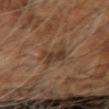{
  "biopsy_status": "not biopsied; imaged during a skin examination",
  "lighting": "cross-polarized",
  "image": {
    "source": "total-body photography crop",
    "field_of_view_mm": 15
  },
  "lesion_size": {
    "long_diameter_mm_approx": 4.0
  },
  "patient": {
    "sex": "male",
    "age_approx": 60
  },
  "site": "arm",
  "automated_metrics": {
    "area_mm2_approx": 7.0,
    "eccentricity": 0.7,
    "shape_asymmetry": 0.4,
    "nevus_likeness_0_100": 0,
    "lesion_detection_confidence_0_100": 90
  }
}